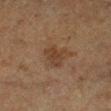Case summary:
• biopsy status: catalogued during a skin exam; not biopsied
• tile lighting: cross-polarized
• location: the right lower leg
• subject: male, roughly 75 years of age
• imaging modality: 15 mm crop, total-body photography
• automated lesion analysis: a footprint of about 6.5 mm², a shape eccentricity near 0.55, and two-axis asymmetry of about 0.4; an average lesion color of about L≈31 a*≈14 b*≈24 (CIELAB), about 6 CIELAB-L* units darker than the surrounding skin, and a normalized border contrast of about 6; a within-lesion color-variation index near 2/10 and peripheral color asymmetry of about 0.5; an automated nevus-likeness rating near 5 out of 100 and a lesion-detection confidence of about 100/100